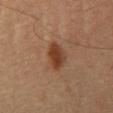Findings:
- biopsy status · total-body-photography surveillance lesion; no biopsy
- lesion size · ≈4 mm
- acquisition · ~15 mm crop, total-body skin-cancer survey
- subject · male, aged around 60
- tile lighting · cross-polarized
- anatomic site · the upper back